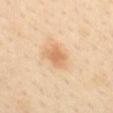  patient:
    sex: female
    age_approx: 40
  lighting: cross-polarized
  site: mid back
  automated_metrics:
    area_mm2_approx: 6.0
    eccentricity: 0.7
    shape_asymmetry: 0.3
    cielab_L: 70
    cielab_a: 21
    cielab_b: 39
    vs_skin_darker_L: 10.0
    vs_skin_contrast_norm: 6.5
    border_irregularity_0_10: 2.5
    color_variation_0_10: 2.5
  image:
    source: total-body photography crop
    field_of_view_mm: 15